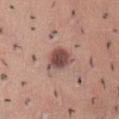No biopsy was performed on this lesion — it was imaged during a full skin examination and was not determined to be concerning.
This is a white-light tile.
A 15 mm close-up tile from a total-body photography series done for melanoma screening.
Measured at roughly 3.5 mm in maximum diameter.
The lesion is located on the lower back.
A female patient aged around 35.